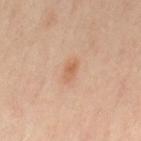workup=catalogued during a skin exam; not biopsied | image source=~15 mm tile from a whole-body skin photo | patient=female, aged approximately 40 | illumination=cross-polarized illumination | body site=the right leg.